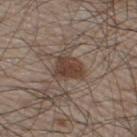{"lesion_size": {"long_diameter_mm_approx": 3.5}, "image": {"source": "total-body photography crop", "field_of_view_mm": 15}, "site": "left lower leg", "lighting": "white-light", "patient": {"sex": "male", "age_approx": 60}}